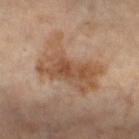Recorded during total-body skin imaging; not selected for excision or biopsy. The lesion is on the right thigh. Imaged with cross-polarized lighting. Longest diameter approximately 7.5 mm. A female patient aged around 75. A region of skin cropped from a whole-body photographic capture, roughly 15 mm wide.